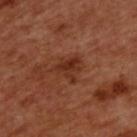Recorded during total-body skin imaging; not selected for excision or biopsy. The subject is a male aged 48–52. A 15 mm crop from a total-body photograph taken for skin-cancer surveillance. An algorithmic analysis of the crop reported roughly 8 lightness units darker than nearby skin and a lesion-to-skin contrast of about 7 (normalized; higher = more distinct). And it measured a nevus-likeness score of about 5/100. Measured at roughly 3 mm in maximum diameter. The lesion is located on the upper back. Captured under cross-polarized illumination.